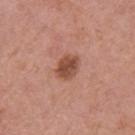Clinical impression:
Recorded during total-body skin imaging; not selected for excision or biopsy.
Background:
Cropped from a whole-body photographic skin survey; the tile spans about 15 mm. Approximately 3.5 mm at its widest. On the right upper arm. Captured under white-light illumination. Automated image analysis of the tile measured a mean CIELAB color near L≈49 a*≈24 b*≈29, roughly 12 lightness units darker than nearby skin, and a normalized lesion–skin contrast near 8.5. The software also gave a classifier nevus-likeness of about 75/100 and a lesion-detection confidence of about 100/100. A female patient aged 48–52.